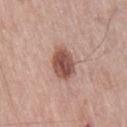Case summary:
– notes · catalogued during a skin exam; not biopsied
– tile lighting · white-light illumination
– diameter · ≈4 mm
– subject · male, approximately 75 years of age
– acquisition · 15 mm crop, total-body photography
– location · the right thigh